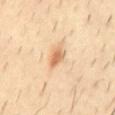Background:
Automated image analysis of the tile measured an area of roughly 4 mm² and a shape eccentricity near 0.85. And it measured a detector confidence of about 100 out of 100 that the crop contains a lesion. The patient is a male aged 38 to 42. A lesion tile, about 15 mm wide, cut from a 3D total-body photograph. On the mid back.